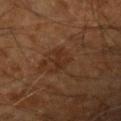Case summary:
• workup — total-body-photography surveillance lesion; no biopsy
• tile lighting — cross-polarized
• patient — male, roughly 60 years of age
• automated metrics — an area of roughly 5 mm², an outline eccentricity of about 0.85 (0 = round, 1 = elongated), and two-axis asymmetry of about 0.45; an automated nevus-likeness rating near 0 out of 100 and a lesion-detection confidence of about 100/100
• body site — the right forearm
• imaging modality — total-body-photography crop, ~15 mm field of view
• lesion size — about 4 mm